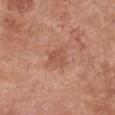Recorded during total-body skin imaging; not selected for excision or biopsy. From the chest. A 15 mm close-up tile from a total-body photography series done for melanoma screening. The lesion-visualizer software estimated an area of roughly 5.5 mm² and an eccentricity of roughly 0.6. And it measured a border-irregularity rating of about 3/10, a color-variation rating of about 2/10, and peripheral color asymmetry of about 0.5. Longest diameter approximately 3 mm. A female patient in their mid-60s. Imaged with white-light lighting.